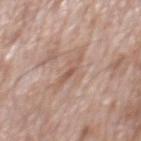follow-up: no biopsy performed (imaged during a skin exam); lesion size: about 3.5 mm; lighting: white-light; anatomic site: the mid back; acquisition: total-body-photography crop, ~15 mm field of view; patient: male, roughly 70 years of age.